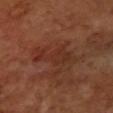{"site": "left forearm", "automated_metrics": {"area_mm2_approx": 10.0, "eccentricity": 0.9, "shape_asymmetry": 0.45, "cielab_L": 30, "cielab_a": 23, "cielab_b": 27, "border_irregularity_0_10": 7.0, "color_variation_0_10": 2.5, "nevus_likeness_0_100": 0, "lesion_detection_confidence_0_100": 100}, "lighting": "cross-polarized", "image": {"source": "total-body photography crop", "field_of_view_mm": 15}, "patient": {"sex": "female", "age_approx": 70}, "lesion_size": {"long_diameter_mm_approx": 6.0}}